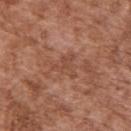Imaged during a routine full-body skin examination; the lesion was not biopsied and no histopathology is available. A male subject aged approximately 75. A region of skin cropped from a whole-body photographic capture, roughly 15 mm wide. The total-body-photography lesion software estimated a mean CIELAB color near L≈47 a*≈23 b*≈29, about 7 CIELAB-L* units darker than the surrounding skin, and a normalized border contrast of about 5. It also reported a border-irregularity rating of about 5.5/10 and peripheral color asymmetry of about 0.5. It also reported an automated nevus-likeness rating near 0 out of 100 and a detector confidence of about 90 out of 100 that the crop contains a lesion. The lesion is located on the upper back. Longest diameter approximately 3.5 mm. Captured under white-light illumination.